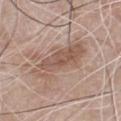Image and clinical context: The lesion is on the chest. Longest diameter approximately 7 mm. The lesion-visualizer software estimated a lesion color around L≈54 a*≈18 b*≈26 in CIELAB, about 10 CIELAB-L* units darker than the surrounding skin, and a lesion-to-skin contrast of about 7 (normalized; higher = more distinct). A male subject about 75 years old. A 15 mm crop from a total-body photograph taken for skin-cancer surveillance. Captured under white-light illumination.